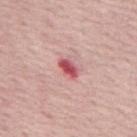Case summary:
– workup: catalogued during a skin exam; not biopsied
– image-analysis metrics: a lesion area of about 3.5 mm² and a shape-asymmetry score of about 0.25 (0 = symmetric); a lesion color around L≈55 a*≈35 b*≈22 in CIELAB, about 15 CIELAB-L* units darker than the surrounding skin, and a normalized lesion–skin contrast near 10
– lesion diameter: ~2.5 mm (longest diameter)
– acquisition: ~15 mm tile from a whole-body skin photo
– tile lighting: white-light illumination
– patient: male, aged approximately 65
– anatomic site: the upper back